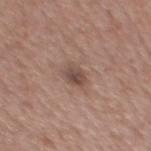The lesion was tiled from a total-body skin photograph and was not biopsied. The subject is a male in their mid-50s. An algorithmic analysis of the crop reported a lesion area of about 4 mm², an outline eccentricity of about 0.6 (0 = round, 1 = elongated), and two-axis asymmetry of about 0.2. The analysis additionally found a border-irregularity rating of about 2/10. And it measured an automated nevus-likeness rating near 35 out of 100 and lesion-presence confidence of about 100/100. Cropped from a whole-body photographic skin survey; the tile spans about 15 mm. Located on the mid back.